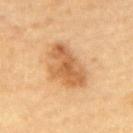Q: Was this lesion biopsied?
A: catalogued during a skin exam; not biopsied
Q: What kind of image is this?
A: ~15 mm tile from a whole-body skin photo
Q: Lesion location?
A: the upper back
Q: What is the lesion's diameter?
A: ≈5.5 mm
Q: Patient demographics?
A: male, in their mid- to late 80s or older
Q: Automated lesion metrics?
A: a lesion area of about 16 mm², an outline eccentricity of about 0.75 (0 = round, 1 = elongated), and a symmetry-axis asymmetry near 0.3; a lesion color around L≈60 a*≈22 b*≈40 in CIELAB, a lesion–skin lightness drop of about 12, and a normalized lesion–skin contrast near 7.5; a classifier nevus-likeness of about 75/100 and lesion-presence confidence of about 100/100